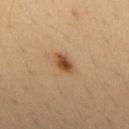The lesion was tiled from a total-body skin photograph and was not biopsied.
The patient is a male in their mid- to late 30s.
Automated image analysis of the tile measured a lesion area of about 5 mm², a shape eccentricity near 0.8, and a shape-asymmetry score of about 0.2 (0 = symmetric). The analysis additionally found a border-irregularity index near 2/10 and internal color variation of about 4 on a 0–10 scale.
Approximately 3 mm at its widest.
On the upper back.
Cropped from a whole-body photographic skin survey; the tile spans about 15 mm.
The tile uses cross-polarized illumination.